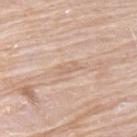<tbp_lesion>
  <biopsy_status>not biopsied; imaged during a skin examination</biopsy_status>
  <automated_metrics>
    <area_mm2_approx>2.5</area_mm2_approx>
    <eccentricity>0.9</eccentricity>
    <shape_asymmetry>0.25</shape_asymmetry>
    <border_irregularity_0_10>3.0</border_irregularity_0_10>
    <color_variation_0_10>0.5</color_variation_0_10>
    <peripheral_color_asymmetry>0.0</peripheral_color_asymmetry>
    <nevus_likeness_0_100>0</nevus_likeness_0_100>
    <lesion_detection_confidence_0_100>65</lesion_detection_confidence_0_100>
  </automated_metrics>
  <image>
    <source>total-body photography crop</source>
    <field_of_view_mm>15</field_of_view_mm>
  </image>
  <patient>
    <sex>male</sex>
    <age_approx>75</age_approx>
  </patient>
  <site>back</site>
  <lesion_size>
    <long_diameter_mm_approx>2.5</long_diameter_mm_approx>
  </lesion_size>
</tbp_lesion>